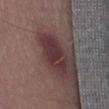Approximately 5.5 mm at its widest. An algorithmic analysis of the crop reported a symmetry-axis asymmetry near 0.25. On the leg. This image is a 15 mm lesion crop taken from a total-body photograph. Imaged with white-light lighting. The subject is a male aged 53 to 57.